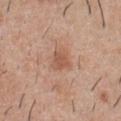  biopsy_status: not biopsied; imaged during a skin examination
  site: chest
  image:
    source: total-body photography crop
    field_of_view_mm: 15
  automated_metrics:
    area_mm2_approx: 5.0
    eccentricity: 0.5
    shape_asymmetry: 0.25
    vs_skin_contrast_norm: 6.0
    lesion_detection_confidence_0_100: 100
  lighting: white-light
  patient:
    sex: male
    age_approx: 30
  lesion_size:
    long_diameter_mm_approx: 2.5